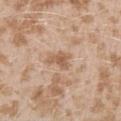Impression: This lesion was catalogued during total-body skin photography and was not selected for biopsy. Acquisition and patient details: Located on the left upper arm. Automated image analysis of the tile measured internal color variation of about 2 on a 0–10 scale and a peripheral color-asymmetry measure near 0.5. The analysis additionally found an automated nevus-likeness rating near 0 out of 100. This image is a 15 mm lesion crop taken from a total-body photograph. A female patient aged 23 to 27. The recorded lesion diameter is about 2.5 mm.